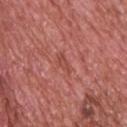The lesion was photographed on a routine skin check and not biopsied; there is no pathology result. A male patient, in their 70s. Approximately 2.5 mm at its widest. Cropped from a total-body skin-imaging series; the visible field is about 15 mm. From the upper back. The lesion-visualizer software estimated about 6 CIELAB-L* units darker than the surrounding skin and a lesion-to-skin contrast of about 5.5 (normalized; higher = more distinct). And it measured a classifier nevus-likeness of about 0/100 and lesion-presence confidence of about 100/100. This is a white-light tile.